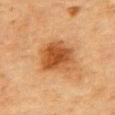No biopsy was performed on this lesion — it was imaged during a full skin examination and was not determined to be concerning. The lesion is located on the chest. The patient is a male aged approximately 85 or older. This is a cross-polarized tile. A close-up tile cropped from a whole-body skin photograph, about 15 mm across.